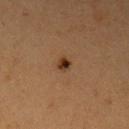biopsy status: catalogued during a skin exam; not biopsied | patient: female, in their 40s | body site: the left upper arm | acquisition: total-body-photography crop, ~15 mm field of view | lighting: cross-polarized.